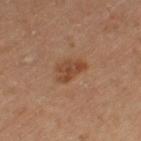The lesion was photographed on a routine skin check and not biopsied; there is no pathology result.
A male subject approximately 65 years of age.
The lesion is located on the left thigh.
A 15 mm close-up extracted from a 3D total-body photography capture.
The tile uses cross-polarized illumination.
About 3.5 mm across.